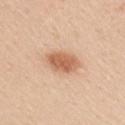Assessment: Captured during whole-body skin photography for melanoma surveillance; the lesion was not biopsied. Background: Automated image analysis of the tile measured a lesion area of about 8.5 mm², an eccentricity of roughly 0.8, and a shape-asymmetry score of about 0.2 (0 = symmetric). And it measured a normalized lesion–skin contrast near 8.5. And it measured a border-irregularity index near 2/10, a color-variation rating of about 3/10, and radial color variation of about 1. And it measured a detector confidence of about 100 out of 100 that the crop contains a lesion. A male patient in their 50s. Imaged with white-light lighting. Located on the right upper arm. A 15 mm close-up extracted from a 3D total-body photography capture. The lesion's longest dimension is about 4 mm.